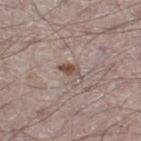* notes: imaged on a skin check; not biopsied
* body site: the left thigh
* automated metrics: a lesion color around L≈50 a*≈16 b*≈23 in CIELAB, about 10 CIELAB-L* units darker than the surrounding skin, and a normalized border contrast of about 8; a border-irregularity rating of about 4.5/10 and radial color variation of about 1.5
* image: total-body-photography crop, ~15 mm field of view
* lesion size: ≈3 mm
* subject: male, aged approximately 50